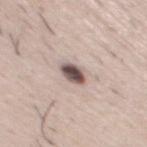Clinical impression:
Recorded during total-body skin imaging; not selected for excision or biopsy.
Context:
A region of skin cropped from a whole-body photographic capture, roughly 15 mm wide. A male patient, about 50 years old. The lesion is located on the chest.